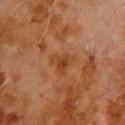{"biopsy_status": "not biopsied; imaged during a skin examination", "lighting": "cross-polarized", "automated_metrics": {"area_mm2_approx": 5.0, "eccentricity": 0.55, "shape_asymmetry": 0.5}, "image": {"source": "total-body photography crop", "field_of_view_mm": 15}, "site": "upper back", "patient": {"sex": "male", "age_approx": 80}, "lesion_size": {"long_diameter_mm_approx": 3.0}}